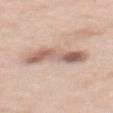biopsy status: imaged on a skin check; not biopsied | image: ~15 mm crop, total-body skin-cancer survey | lighting: white-light illumination | lesion diameter: about 5.5 mm | TBP lesion metrics: an eccentricity of roughly 0.9 and a shape-asymmetry score of about 0.3 (0 = symmetric); an average lesion color of about L≈61 a*≈18 b*≈26 (CIELAB), about 13 CIELAB-L* units darker than the surrounding skin, and a normalized lesion–skin contrast near 8; a border-irregularity rating of about 4/10, a within-lesion color-variation index near 7.5/10, and peripheral color asymmetry of about 2; a nevus-likeness score of about 40/100 and a lesion-detection confidence of about 100/100 | subject: male, aged around 60 | location: the mid back.